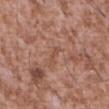A 15 mm close-up tile from a total-body photography series done for melanoma screening. Imaged with white-light lighting. A male subject, about 45 years old. Longest diameter approximately 2.5 mm. The lesion is located on the abdomen.